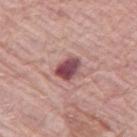The lesion was photographed on a routine skin check and not biopsied; there is no pathology result. Approximately 3 mm at its widest. Captured under white-light illumination. A female subject, aged 68 to 72. On the left thigh. Cropped from a whole-body photographic skin survey; the tile spans about 15 mm.